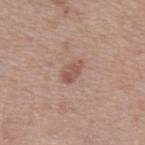This lesion was catalogued during total-body skin photography and was not selected for biopsy. A close-up tile cropped from a whole-body skin photograph, about 15 mm across. A male patient, approximately 45 years of age. The lesion's longest dimension is about 3 mm. The tile uses white-light illumination. From the arm.